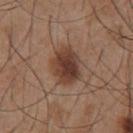Part of a total-body skin-imaging series; this lesion was reviewed on a skin check and was not flagged for biopsy.
The subject is a male aged approximately 55.
The total-body-photography lesion software estimated a lesion area of about 11 mm², an eccentricity of roughly 0.65, and a symmetry-axis asymmetry near 0.15. And it measured a lesion–skin lightness drop of about 12. And it measured border irregularity of about 2 on a 0–10 scale, internal color variation of about 4.5 on a 0–10 scale, and peripheral color asymmetry of about 1.5. The software also gave a classifier nevus-likeness of about 95/100 and a lesion-detection confidence of about 100/100.
The tile uses white-light illumination.
A lesion tile, about 15 mm wide, cut from a 3D total-body photograph.
The lesion is on the chest.